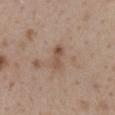Captured during whole-body skin photography for melanoma surveillance; the lesion was not biopsied. Longest diameter approximately 3 mm. The lesion is on the mid back. The total-body-photography lesion software estimated a border-irregularity index near 2.5/10, a within-lesion color-variation index near 4/10, and a peripheral color-asymmetry measure near 1.5. It also reported a classifier nevus-likeness of about 0/100 and a lesion-detection confidence of about 100/100. A 15 mm close-up extracted from a 3D total-body photography capture. A female subject in their mid-40s. The tile uses white-light illumination.